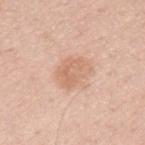workup: catalogued during a skin exam; not biopsied
acquisition: 15 mm crop, total-body photography
body site: the left upper arm
size: ~4 mm (longest diameter)
patient: male, aged 63 to 67
automated lesion analysis: an area of roughly 9.5 mm², a shape eccentricity near 0.65, and a shape-asymmetry score of about 0.2 (0 = symmetric); a mean CIELAB color near L≈66 a*≈21 b*≈32 and about 8 CIELAB-L* units darker than the surrounding skin; a nevus-likeness score of about 5/100 and a lesion-detection confidence of about 100/100